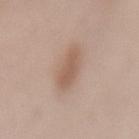Assessment: Captured during whole-body skin photography for melanoma surveillance; the lesion was not biopsied. Image and clinical context: Imaged with white-light lighting. Located on the mid back. Automated tile analysis of the lesion measured a shape eccentricity near 0.7 and two-axis asymmetry of about 0.25. The software also gave an average lesion color of about L≈57 a*≈17 b*≈28 (CIELAB) and roughly 9 lightness units darker than nearby skin. And it measured border irregularity of about 2.5 on a 0–10 scale, internal color variation of about 1.5 on a 0–10 scale, and a peripheral color-asymmetry measure near 0.5. The software also gave an automated nevus-likeness rating near 70 out of 100. A 15 mm close-up tile from a total-body photography series done for melanoma screening. The patient is a female aged 48 to 52.